A lesion tile, about 15 mm wide, cut from a 3D total-body photograph; the lesion is on the chest; the patient is a male aged 38 to 42; approximately 6.5 mm at its widest.
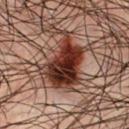Conclusion: Histopathologically confirmed as a melanoma in situ, superficial spreading type (malignant).